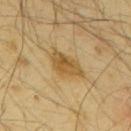Clinical impression:
This lesion was catalogued during total-body skin photography and was not selected for biopsy.
Acquisition and patient details:
The subject is a male roughly 65 years of age. Approximately 5.5 mm at its widest. Captured under cross-polarized illumination. The lesion-visualizer software estimated an area of roughly 9 mm². The software also gave a classifier nevus-likeness of about 0/100 and a lesion-detection confidence of about 90/100. On the mid back. A region of skin cropped from a whole-body photographic capture, roughly 15 mm wide.